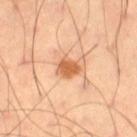follow-up = catalogued during a skin exam; not biopsied | tile lighting = cross-polarized illumination | anatomic site = the right thigh | image-analysis metrics = a normalized border contrast of about 9; internal color variation of about 2 on a 0–10 scale and a peripheral color-asymmetry measure near 0.5; an automated nevus-likeness rating near 90 out of 100 | patient = male, aged around 55 | imaging modality = ~15 mm tile from a whole-body skin photo | size = ≈2.5 mm.